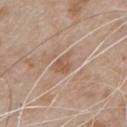Q: Was a biopsy performed?
A: catalogued during a skin exam; not biopsied
Q: Patient demographics?
A: male, roughly 80 years of age
Q: How was this image acquired?
A: ~15 mm crop, total-body skin-cancer survey
Q: Lesion size?
A: ≈3 mm
Q: Lesion location?
A: the chest
Q: What did automated image analysis measure?
A: an area of roughly 5 mm² and an eccentricity of roughly 0.7; a lesion–skin lightness drop of about 7 and a normalized border contrast of about 5.5; a border-irregularity index near 3.5/10 and peripheral color asymmetry of about 0.5
Q: What lighting was used for the tile?
A: white-light